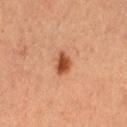Captured during whole-body skin photography for melanoma surveillance; the lesion was not biopsied.
On the left thigh.
Automated image analysis of the tile measured a footprint of about 4 mm² and a shape eccentricity near 0.6. And it measured a within-lesion color-variation index near 3/10 and radial color variation of about 1. The software also gave an automated nevus-likeness rating near 100 out of 100.
The recorded lesion diameter is about 2.5 mm.
The patient is a female aged approximately 40.
A roughly 15 mm field-of-view crop from a total-body skin photograph.
This is a cross-polarized tile.